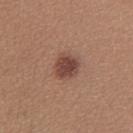This lesion was catalogued during total-body skin photography and was not selected for biopsy. The lesion's longest dimension is about 3.5 mm. The subject is a female roughly 35 years of age. A close-up tile cropped from a whole-body skin photograph, about 15 mm across. Located on the upper back.